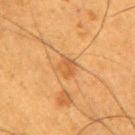{
  "biopsy_status": "not biopsied; imaged during a skin examination",
  "site": "right upper arm",
  "image": {
    "source": "total-body photography crop",
    "field_of_view_mm": 15
  },
  "patient": {
    "sex": "male",
    "age_approx": 50
  },
  "lesion_size": {
    "long_diameter_mm_approx": 2.5
  },
  "lighting": "cross-polarized",
  "automated_metrics": {
    "area_mm2_approx": 4.0,
    "eccentricity": 0.55,
    "shape_asymmetry": 0.3,
    "cielab_L": 46,
    "cielab_a": 21,
    "cielab_b": 37,
    "vs_skin_darker_L": 7.0,
    "border_irregularity_0_10": 3.0,
    "color_variation_0_10": 2.0,
    "peripheral_color_asymmetry": 0.5
  }
}